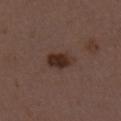| feature | finding |
|---|---|
| biopsy status | total-body-photography surveillance lesion; no biopsy |
| image source | ~15 mm tile from a whole-body skin photo |
| subject | female, about 50 years old |
| location | the front of the torso |